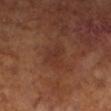notes: no biopsy performed (imaged during a skin exam)
automated lesion analysis: a mean CIELAB color near L≈33 a*≈22 b*≈27, a lesion–skin lightness drop of about 5, and a lesion-to-skin contrast of about 5 (normalized; higher = more distinct)
patient: male, aged around 65
lighting: cross-polarized illumination
body site: the left lower leg
image: total-body-photography crop, ~15 mm field of view